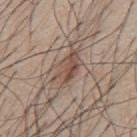Recorded during total-body skin imaging; not selected for excision or biopsy. About 5 mm across. Cropped from a whole-body photographic skin survey; the tile spans about 15 mm. The lesion is located on the mid back. The subject is a male approximately 45 years of age. An algorithmic analysis of the crop reported an area of roughly 8.5 mm² and an outline eccentricity of about 0.85 (0 = round, 1 = elongated). The software also gave a border-irregularity rating of about 4.5/10, a within-lesion color-variation index near 4.5/10, and peripheral color asymmetry of about 1.5. And it measured a classifier nevus-likeness of about 35/100 and a lesion-detection confidence of about 95/100. Captured under white-light illumination.